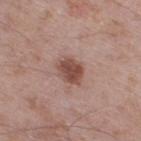No biopsy was performed on this lesion — it was imaged during a full skin examination and was not determined to be concerning. This image is a 15 mm lesion crop taken from a total-body photograph. Located on the left thigh. A male subject, aged 58 to 62. An algorithmic analysis of the crop reported an area of roughly 7 mm² and two-axis asymmetry of about 0.2. And it measured an automated nevus-likeness rating near 90 out of 100. The tile uses white-light illumination.